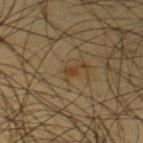illumination — cross-polarized
diameter — about 1.5 mm
acquisition — total-body-photography crop, ~15 mm field of view
anatomic site — the upper back
subject — male, aged 43 to 47